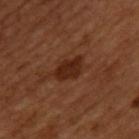  biopsy_status: not biopsied; imaged during a skin examination
  lesion_size:
    long_diameter_mm_approx: 4.0
  site: upper back
  automated_metrics:
    cielab_L: 26
    cielab_a: 22
    cielab_b: 29
    vs_skin_darker_L: 9.0
    vs_skin_contrast_norm: 9.0
    border_irregularity_0_10: 3.0
    color_variation_0_10: 2.5
    peripheral_color_asymmetry: 1.0
  lighting: cross-polarized
  image:
    source: total-body photography crop
    field_of_view_mm: 15
  patient:
    sex: male
    age_approx: 50